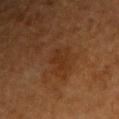notes: catalogued during a skin exam; not biopsied | acquisition: ~15 mm tile from a whole-body skin photo | patient: male, roughly 65 years of age | lesion size: about 3 mm | site: the right upper arm | illumination: cross-polarized illumination.